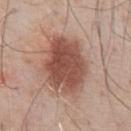{"biopsy_status": "not biopsied; imaged during a skin examination", "lighting": "white-light", "image": {"source": "total-body photography crop", "field_of_view_mm": 15}, "lesion_size": {"long_diameter_mm_approx": 6.0}, "patient": {"sex": "male", "age_approx": 60}, "site": "abdomen"}